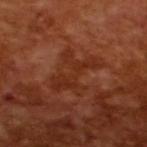biopsy status=imaged on a skin check; not biopsied
subject=male, aged 63 to 67
diameter=about 6 mm
illumination=cross-polarized
image source=15 mm crop, total-body photography